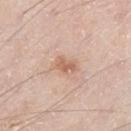The lesion was tiled from a total-body skin photograph and was not biopsied. A male subject, roughly 60 years of age. The lesion's longest dimension is about 2.5 mm. Located on the leg. A 15 mm close-up tile from a total-body photography series done for melanoma screening.